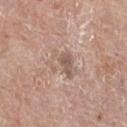The lesion was photographed on a routine skin check and not biopsied; there is no pathology result.